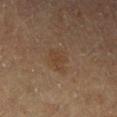{"biopsy_status": "not biopsied; imaged during a skin examination", "site": "left lower leg", "lesion_size": {"long_diameter_mm_approx": 3.0}, "patient": {"sex": "male", "age_approx": "85+"}, "image": {"source": "total-body photography crop", "field_of_view_mm": 15}}